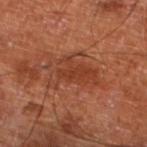| feature | finding |
|---|---|
| notes | no biopsy performed (imaged during a skin exam) |
| imaging modality | ~15 mm crop, total-body skin-cancer survey |
| tile lighting | cross-polarized illumination |
| automated metrics | a footprint of about 11 mm² and an eccentricity of roughly 0.85; a border-irregularity index near 6/10, a color-variation rating of about 3/10, and a peripheral color-asymmetry measure near 1; an automated nevus-likeness rating near 25 out of 100 |
| diameter | ~5 mm (longest diameter) |
| anatomic site | the left lower leg |
| subject | roughly 65 years of age |